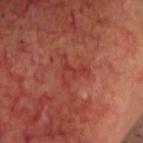biopsy_status: not biopsied; imaged during a skin examination
automated_metrics:
  area_mm2_approx: 6.0
  shape_asymmetry: 0.75
  cielab_L: 39
  cielab_a: 33
  cielab_b: 28
  vs_skin_contrast_norm: 5.0
  border_irregularity_0_10: 8.5
  color_variation_0_10: 3.0
  peripheral_color_asymmetry: 1.0
lesion_size:
  long_diameter_mm_approx: 4.0
lighting: cross-polarized
patient:
  sex: male
  age_approx: 65
site: head or neck
image:
  source: total-body photography crop
  field_of_view_mm: 15